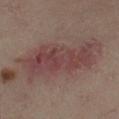The lesion is on the right lower leg. The tile uses cross-polarized illumination. A 15 mm close-up tile from a total-body photography series done for melanoma screening. A female subject, aged 33–37. Measured at roughly 10.5 mm in maximum diameter.